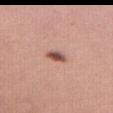workup: total-body-photography surveillance lesion; no biopsy
image-analysis metrics: a mean CIELAB color near L≈52 a*≈22 b*≈25, a lesion–skin lightness drop of about 15, and a lesion-to-skin contrast of about 10 (normalized; higher = more distinct)
location: the left thigh
subject: female, aged 38–42
image: total-body-photography crop, ~15 mm field of view